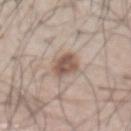The lesion was photographed on a routine skin check and not biopsied; there is no pathology result.
The lesion is on the chest.
A male subject, in their mid-60s.
Captured under white-light illumination.
A roughly 15 mm field-of-view crop from a total-body skin photograph.
The lesion-visualizer software estimated border irregularity of about 2 on a 0–10 scale and a color-variation rating of about 4.5/10.
Measured at roughly 3.5 mm in maximum diameter.